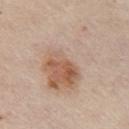Q: Was a biopsy performed?
A: total-body-photography surveillance lesion; no biopsy
Q: How was this image acquired?
A: total-body-photography crop, ~15 mm field of view
Q: What is the lesion's diameter?
A: about 10.5 mm
Q: Illumination type?
A: white-light
Q: Lesion location?
A: the chest
Q: What are the patient's age and sex?
A: female, in their 70s